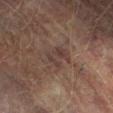Case summary:
* follow-up: catalogued during a skin exam; not biopsied
* lesion diameter: ≈3 mm
* patient: male, aged around 75
* tile lighting: cross-polarized illumination
* image: total-body-photography crop, ~15 mm field of view
* automated lesion analysis: an area of roughly 5.5 mm², an eccentricity of roughly 0.7, and a symmetry-axis asymmetry near 0.3; a nevus-likeness score of about 0/100
* anatomic site: the right lower leg